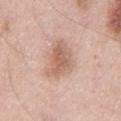Clinical impression: Part of a total-body skin-imaging series; this lesion was reviewed on a skin check and was not flagged for biopsy. Acquisition and patient details: A male subject in their mid- to late 50s. This is a white-light tile. A roughly 15 mm field-of-view crop from a total-body skin photograph. On the chest.